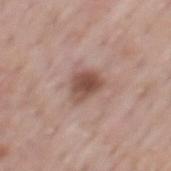{
  "biopsy_status": "not biopsied; imaged during a skin examination",
  "patient": {
    "sex": "male",
    "age_approx": 55
  },
  "site": "mid back",
  "automated_metrics": {
    "vs_skin_darker_L": 12.0,
    "vs_skin_contrast_norm": 9.0,
    "border_irregularity_0_10": 2.5,
    "color_variation_0_10": 4.5,
    "peripheral_color_asymmetry": 1.5,
    "lesion_detection_confidence_0_100": 100
  },
  "image": {
    "source": "total-body photography crop",
    "field_of_view_mm": 15
  },
  "lesion_size": {
    "long_diameter_mm_approx": 3.5
  }
}